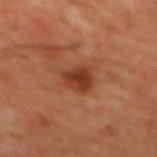Clinical impression: This lesion was catalogued during total-body skin photography and was not selected for biopsy. Context: From the chest. A 15 mm close-up extracted from a 3D total-body photography capture. Imaged with cross-polarized lighting. A male subject in their 60s.